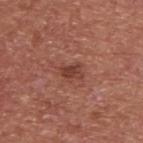Clinical impression: Recorded during total-body skin imaging; not selected for excision or biopsy. Image and clinical context: A 15 mm crop from a total-body photograph taken for skin-cancer surveillance. Longest diameter approximately 2.5 mm. The tile uses white-light illumination. A male patient, aged 63 to 67. Located on the upper back.